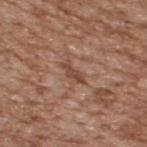Imaged during a routine full-body skin examination; the lesion was not biopsied and no histopathology is available.
Approximately 3 mm at its widest.
From the upper back.
A 15 mm crop from a total-body photograph taken for skin-cancer surveillance.
The tile uses white-light illumination.
A male patient, aged approximately 65.